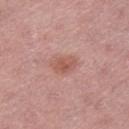No biopsy was performed on this lesion — it was imaged during a full skin examination and was not determined to be concerning. The patient is a female in their mid-40s. The lesion is located on the right thigh. Captured under white-light illumination. About 3 mm across. Automated tile analysis of the lesion measured a border-irregularity index near 2/10, internal color variation of about 2.5 on a 0–10 scale, and a peripheral color-asymmetry measure near 0.5. And it measured lesion-presence confidence of about 100/100. A region of skin cropped from a whole-body photographic capture, roughly 15 mm wide.